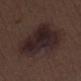This lesion was catalogued during total-body skin photography and was not selected for biopsy. A roughly 15 mm field-of-view crop from a total-body skin photograph. The lesion is on the leg. The total-body-photography lesion software estimated a mean CIELAB color near L≈23 a*≈14 b*≈14, about 8 CIELAB-L* units darker than the surrounding skin, and a lesion-to-skin contrast of about 10.5 (normalized; higher = more distinct). The analysis additionally found a classifier nevus-likeness of about 45/100 and a lesion-detection confidence of about 100/100. The patient is a male in their 70s.